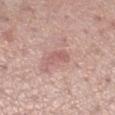{"biopsy_status": "not biopsied; imaged during a skin examination", "automated_metrics": {"eccentricity": 0.8, "shape_asymmetry": 0.3, "border_irregularity_0_10": 3.0, "color_variation_0_10": 2.5, "peripheral_color_asymmetry": 1.0, "nevus_likeness_0_100": 10, "lesion_detection_confidence_0_100": 100}, "lighting": "white-light", "patient": {"sex": "female", "age_approx": 60}, "site": "right lower leg", "image": {"source": "total-body photography crop", "field_of_view_mm": 15}}